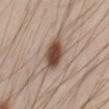Findings:
– follow-up · catalogued during a skin exam; not biopsied
– subject · male, approximately 35 years of age
– lighting · white-light illumination
– lesion diameter · ~5 mm (longest diameter)
– image source · total-body-photography crop, ~15 mm field of view
– location · the back
– automated metrics · a footprint of about 10 mm², an outline eccentricity of about 0.8 (0 = round, 1 = elongated), and two-axis asymmetry of about 0.2; an average lesion color of about L≈49 a*≈17 b*≈25 (CIELAB), about 16 CIELAB-L* units darker than the surrounding skin, and a normalized lesion–skin contrast near 11; internal color variation of about 5 on a 0–10 scale and peripheral color asymmetry of about 1.5